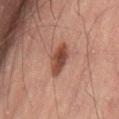Q: Was a biopsy performed?
A: total-body-photography surveillance lesion; no biopsy
Q: What are the patient's age and sex?
A: male, roughly 50 years of age
Q: Lesion location?
A: the lower back
Q: What kind of image is this?
A: ~15 mm crop, total-body skin-cancer survey